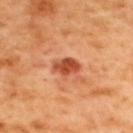The lesion was tiled from a total-body skin photograph and was not biopsied.
A lesion tile, about 15 mm wide, cut from a 3D total-body photograph.
The lesion is on the upper back.
A male subject, in their 50s.
The lesion-visualizer software estimated a footprint of about 5 mm², an outline eccentricity of about 0.8 (0 = round, 1 = elongated), and a shape-asymmetry score of about 0.25 (0 = symmetric). It also reported a lesion-to-skin contrast of about 9.5 (normalized; higher = more distinct). And it measured a classifier nevus-likeness of about 50/100.